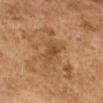<record>
<biopsy_status>not biopsied; imaged during a skin examination</biopsy_status>
<automated_metrics>
  <area_mm2_approx>5.5</area_mm2_approx>
  <shape_asymmetry>0.35</shape_asymmetry>
  <border_irregularity_0_10>4.0</border_irregularity_0_10>
  <peripheral_color_asymmetry>0.5</peripheral_color_asymmetry>
  <nevus_likeness_0_100>0</nevus_likeness_0_100>
  <lesion_detection_confidence_0_100>95</lesion_detection_confidence_0_100>
</automated_metrics>
<patient>
  <sex>female</sex>
  <age_approx>60</age_approx>
</patient>
<site>left upper arm</site>
<lesion_size>
  <long_diameter_mm_approx>4.0</long_diameter_mm_approx>
</lesion_size>
<lighting>cross-polarized</lighting>
<image>
  <source>total-body photography crop</source>
  <field_of_view_mm>15</field_of_view_mm>
</image>
</record>